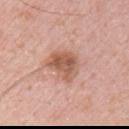The lesion was photographed on a routine skin check and not biopsied; there is no pathology result. The lesion's longest dimension is about 4 mm. On the right upper arm. The tile uses white-light illumination. The lesion-visualizer software estimated internal color variation of about 5 on a 0–10 scale and radial color variation of about 1.5. It also reported a lesion-detection confidence of about 100/100. A 15 mm close-up extracted from a 3D total-body photography capture. The patient is a male roughly 50 years of age.